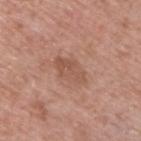No biopsy was performed on this lesion — it was imaged during a full skin examination and was not determined to be concerning.
A 15 mm close-up extracted from a 3D total-body photography capture.
A male patient aged around 40.
Located on the upper back.
Automated image analysis of the tile measured a footprint of about 8 mm², a shape eccentricity near 0.85, and two-axis asymmetry of about 0.15. The software also gave a mean CIELAB color near L≈53 a*≈22 b*≈28, about 7 CIELAB-L* units darker than the surrounding skin, and a normalized border contrast of about 5.5.
The tile uses white-light illumination.